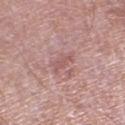Imaged during a routine full-body skin examination; the lesion was not biopsied and no histopathology is available.
The lesion is on the right lower leg.
Longest diameter approximately 3 mm.
Cropped from a whole-body photographic skin survey; the tile spans about 15 mm.
The total-body-photography lesion software estimated a border-irregularity rating of about 4/10, a color-variation rating of about 1.5/10, and radial color variation of about 0.5. It also reported a classifier nevus-likeness of about 0/100.
The subject is a male approximately 65 years of age.